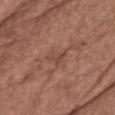Clinical impression:
Recorded during total-body skin imaging; not selected for excision or biopsy.
Context:
Automated tile analysis of the lesion measured a mean CIELAB color near L≈47 a*≈22 b*≈27 and about 6 CIELAB-L* units darker than the surrounding skin. The analysis additionally found border irregularity of about 4 on a 0–10 scale, a color-variation rating of about 3/10, and peripheral color asymmetry of about 1. It also reported a classifier nevus-likeness of about 0/100 and lesion-presence confidence of about 90/100. A close-up tile cropped from a whole-body skin photograph, about 15 mm across. From the chest. The lesion's longest dimension is about 3 mm. Imaged with white-light lighting. A female patient, aged approximately 65.